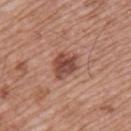Clinical impression: The lesion was tiled from a total-body skin photograph and was not biopsied. Background: Imaged with white-light lighting. The patient is a male aged around 65. The recorded lesion diameter is about 4 mm. From the upper back. Automated image analysis of the tile measured a lesion color around L≈48 a*≈24 b*≈27 in CIELAB and a normalized border contrast of about 9. The software also gave a border-irregularity index near 2.5/10 and a peripheral color-asymmetry measure near 2. Cropped from a total-body skin-imaging series; the visible field is about 15 mm.